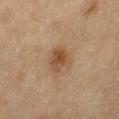Findings:
– follow-up — imaged on a skin check; not biopsied
– site — the left thigh
– diameter — ≈3 mm
– image source — total-body-photography crop, ~15 mm field of view
– automated metrics — a footprint of about 5.5 mm² and two-axis asymmetry of about 0.2; a lesion color around L≈44 a*≈17 b*≈32 in CIELAB, roughly 9 lightness units darker than nearby skin, and a normalized border contrast of about 8; border irregularity of about 2 on a 0–10 scale and radial color variation of about 1.5
– illumination — cross-polarized
– subject — female, aged 58–62